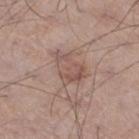Q: Was this lesion biopsied?
A: catalogued during a skin exam; not biopsied
Q: Illumination type?
A: white-light illumination
Q: What kind of image is this?
A: total-body-photography crop, ~15 mm field of view
Q: Who is the patient?
A: male, aged around 55
Q: How large is the lesion?
A: about 4.5 mm
Q: Lesion location?
A: the right thigh